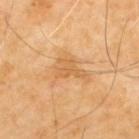workup: imaged on a skin check; not biopsied | illumination: cross-polarized illumination | image-analysis metrics: a lesion area of about 5.5 mm² and an outline eccentricity of about 0.8 (0 = round, 1 = elongated); roughly 8 lightness units darker than nearby skin and a lesion-to-skin contrast of about 5.5 (normalized; higher = more distinct); a border-irregularity rating of about 6/10, a color-variation rating of about 2/10, and a peripheral color-asymmetry measure near 1; lesion-presence confidence of about 100/100 | body site: the upper back | size: ≈3.5 mm | patient: male, about 70 years old | acquisition: ~15 mm crop, total-body skin-cancer survey.